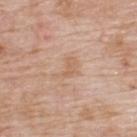Clinical impression:
The lesion was tiled from a total-body skin photograph and was not biopsied.
Image and clinical context:
The tile uses white-light illumination. A male subject aged 58 to 62. A close-up tile cropped from a whole-body skin photograph, about 15 mm across. On the upper back. An algorithmic analysis of the crop reported a lesion area of about 4 mm², an outline eccentricity of about 0.8 (0 = round, 1 = elongated), and a shape-asymmetry score of about 0.55 (0 = symmetric). It also reported border irregularity of about 6 on a 0–10 scale, internal color variation of about 1 on a 0–10 scale, and a peripheral color-asymmetry measure near 0.5. Approximately 3.5 mm at its widest.